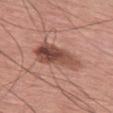Context: An algorithmic analysis of the crop reported an average lesion color of about L≈49 a*≈23 b*≈26 (CIELAB), a lesion–skin lightness drop of about 13, and a normalized lesion–skin contrast near 9.5. The software also gave an automated nevus-likeness rating near 20 out of 100 and a detector confidence of about 100 out of 100 that the crop contains a lesion. This is a white-light tile. A male patient aged approximately 75. Approximately 6.5 mm at its widest. A region of skin cropped from a whole-body photographic capture, roughly 15 mm wide. The lesion is on the left thigh. Conclusion: The lesion was biopsied, and histopathology showed a melanoma in situ.